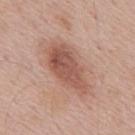On the mid back.
The patient is a male about 55 years old.
Imaged with white-light lighting.
A region of skin cropped from a whole-body photographic capture, roughly 15 mm wide.
Approximately 7 mm at its widest.